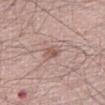Assessment: No biopsy was performed on this lesion — it was imaged during a full skin examination and was not determined to be concerning. Background: Cropped from a whole-body photographic skin survey; the tile spans about 15 mm. A male subject aged approximately 65. The tile uses white-light illumination. On the right thigh.